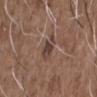Acquisition and patient details: About 3 mm across. A male patient in their 50s. A close-up tile cropped from a whole-body skin photograph, about 15 mm across. Imaged with white-light lighting. On the front of the torso.